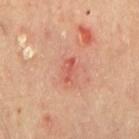Recorded during total-body skin imaging; not selected for excision or biopsy. On the mid back. Imaged with cross-polarized lighting. A male subject, aged 63 to 67. A region of skin cropped from a whole-body photographic capture, roughly 15 mm wide. Approximately 2.5 mm at its widest.